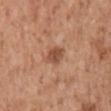{
  "biopsy_status": "not biopsied; imaged during a skin examination",
  "lesion_size": {
    "long_diameter_mm_approx": 3.0
  },
  "automated_metrics": {
    "area_mm2_approx": 5.0,
    "eccentricity": 0.75,
    "shape_asymmetry": 0.25,
    "cielab_L": 51,
    "cielab_a": 23,
    "cielab_b": 33,
    "border_irregularity_0_10": 2.0,
    "color_variation_0_10": 2.5,
    "nevus_likeness_0_100": 65,
    "lesion_detection_confidence_0_100": 100
  },
  "patient": {
    "sex": "male",
    "age_approx": 60
  },
  "site": "mid back",
  "image": {
    "source": "total-body photography crop",
    "field_of_view_mm": 15
  }
}